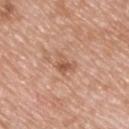The lesion was photographed on a routine skin check and not biopsied; there is no pathology result. The total-body-photography lesion software estimated a lesion area of about 4 mm², a shape eccentricity near 0.65, and a shape-asymmetry score of about 0.55 (0 = symmetric). The analysis additionally found an average lesion color of about L≈56 a*≈22 b*≈32 (CIELAB), a lesion–skin lightness drop of about 9, and a normalized border contrast of about 6.5. The software also gave a nevus-likeness score of about 15/100 and a detector confidence of about 100 out of 100 that the crop contains a lesion. A 15 mm crop from a total-body photograph taken for skin-cancer surveillance. A male subject, aged around 50. Captured under white-light illumination. Measured at roughly 3 mm in maximum diameter. The lesion is on the upper back.